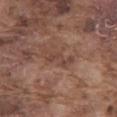follow-up: catalogued during a skin exam; not biopsied | subject: male, in their mid-70s | diameter: ~4 mm (longest diameter) | anatomic site: the abdomen | TBP lesion metrics: a lesion area of about 4.5 mm² and an eccentricity of roughly 0.9 | image: total-body-photography crop, ~15 mm field of view | illumination: white-light illumination.